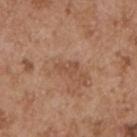No biopsy was performed on this lesion — it was imaged during a full skin examination and was not determined to be concerning.
Automated image analysis of the tile measured an area of roughly 3 mm², an outline eccentricity of about 0.85 (0 = round, 1 = elongated), and two-axis asymmetry of about 0.55. The analysis additionally found a nevus-likeness score of about 0/100.
Cropped from a whole-body photographic skin survey; the tile spans about 15 mm.
Measured at roughly 2.5 mm in maximum diameter.
Imaged with white-light lighting.
A male subject about 55 years old.
On the left upper arm.